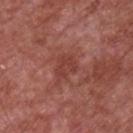On the chest.
A male subject roughly 65 years of age.
A roughly 15 mm field-of-view crop from a total-body skin photograph.
Longest diameter approximately 3 mm.